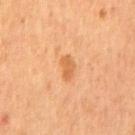The lesion was photographed on a routine skin check and not biopsied; there is no pathology result. On the front of the torso. Imaged with cross-polarized lighting. This image is a 15 mm lesion crop taken from a total-body photograph. An algorithmic analysis of the crop reported a lesion color around L≈58 a*≈24 b*≈41 in CIELAB, roughly 9 lightness units darker than nearby skin, and a normalized border contrast of about 6.5. And it measured a border-irregularity rating of about 3/10, a within-lesion color-variation index near 2.5/10, and peripheral color asymmetry of about 1. The subject is a male aged approximately 55. The lesion's longest dimension is about 3 mm.